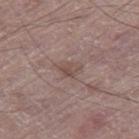| field | value |
|---|---|
| follow-up | no biopsy performed (imaged during a skin exam) |
| body site | the right thigh |
| lesion diameter | ≈2.5 mm |
| image source | 15 mm crop, total-body photography |
| patient | male, in their mid-60s |